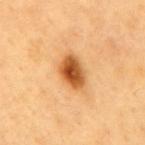lesion size = ≈4 mm
subject = male, approximately 60 years of age
lighting = cross-polarized
location = the mid back
image = 15 mm crop, total-body photography